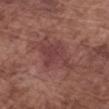The lesion was photographed on a routine skin check and not biopsied; there is no pathology result.
Imaged with white-light lighting.
The total-body-photography lesion software estimated an eccentricity of roughly 0.85. The software also gave a detector confidence of about 100 out of 100 that the crop contains a lesion.
Longest diameter approximately 6.5 mm.
A 15 mm close-up tile from a total-body photography series done for melanoma screening.
The lesion is on the right forearm.
A male patient, aged around 75.